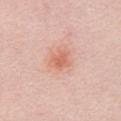Q: Was this lesion biopsied?
A: catalogued during a skin exam; not biopsied
Q: Patient demographics?
A: male, aged 28 to 32
Q: Lesion location?
A: the right upper arm
Q: What did automated image analysis measure?
A: a shape-asymmetry score of about 0.25 (0 = symmetric); border irregularity of about 3 on a 0–10 scale, internal color variation of about 2.5 on a 0–10 scale, and a peripheral color-asymmetry measure near 1
Q: What is the imaging modality?
A: ~15 mm tile from a whole-body skin photo
Q: What is the lesion's diameter?
A: ~3 mm (longest diameter)